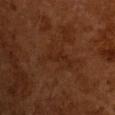<case>
  <biopsy_status>not biopsied; imaged during a skin examination</biopsy_status>
  <image>
    <source>total-body photography crop</source>
    <field_of_view_mm>15</field_of_view_mm>
  </image>
  <patient>
    <sex>male</sex>
    <age_approx>65</age_approx>
  </patient>
  <lesion_size>
    <long_diameter_mm_approx>3.0</long_diameter_mm_approx>
  </lesion_size>
</case>